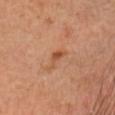Q: Was this lesion biopsied?
A: no biopsy performed (imaged during a skin exam)
Q: What did automated image analysis measure?
A: a footprint of about 3 mm², an eccentricity of roughly 0.9, and two-axis asymmetry of about 0.5; a peripheral color-asymmetry measure near 0; an automated nevus-likeness rating near 15 out of 100 and a detector confidence of about 100 out of 100 that the crop contains a lesion
Q: How was this image acquired?
A: ~15 mm crop, total-body skin-cancer survey
Q: How was the tile lit?
A: cross-polarized illumination
Q: What is the lesion's diameter?
A: about 3 mm
Q: What are the patient's age and sex?
A: female, aged 38–42
Q: Lesion location?
A: the head or neck